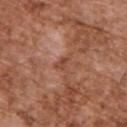{
  "biopsy_status": "not biopsied; imaged during a skin examination",
  "lesion_size": {
    "long_diameter_mm_approx": 3.0
  },
  "image": {
    "source": "total-body photography crop",
    "field_of_view_mm": 15
  },
  "patient": {
    "sex": "male",
    "age_approx": 75
  },
  "site": "upper back"
}